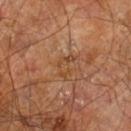This lesion was catalogued during total-body skin photography and was not selected for biopsy.
Captured under cross-polarized illumination.
Longest diameter approximately 3 mm.
A 15 mm close-up extracted from a 3D total-body photography capture.
From the right leg.
The patient is a male in their 60s.
An algorithmic analysis of the crop reported a border-irregularity rating of about 5/10.